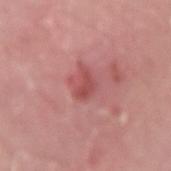This lesion was catalogued during total-body skin photography and was not selected for biopsy. Located on the arm. A female subject, in their 50s. This is a white-light tile. A lesion tile, about 15 mm wide, cut from a 3D total-body photograph.